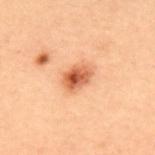{
  "biopsy_status": "not biopsied; imaged during a skin examination",
  "site": "upper back",
  "image": {
    "source": "total-body photography crop",
    "field_of_view_mm": 15
  },
  "patient": {
    "sex": "male",
    "age_approx": 55
  }
}